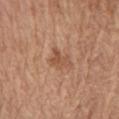biopsy status: total-body-photography surveillance lesion; no biopsy
image source: ~15 mm tile from a whole-body skin photo
subject: male, in their mid-70s
location: the chest